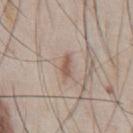Q: Was a biopsy performed?
A: catalogued during a skin exam; not biopsied
Q: How was the tile lit?
A: white-light illumination
Q: What are the patient's age and sex?
A: male, aged around 45
Q: Lesion size?
A: ~2.5 mm (longest diameter)
Q: What did automated image analysis measure?
A: a classifier nevus-likeness of about 5/100 and a lesion-detection confidence of about 100/100
Q: What is the anatomic site?
A: the chest
Q: What is the imaging modality?
A: 15 mm crop, total-body photography